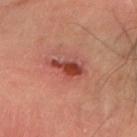Assessment:
This lesion was catalogued during total-body skin photography and was not selected for biopsy.
Image and clinical context:
A male subject in their mid-40s. On the right forearm. A 15 mm close-up tile from a total-body photography series done for melanoma screening. Captured under cross-polarized illumination. Measured at roughly 4.5 mm in maximum diameter.